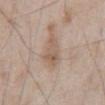Notes:
* workup — catalogued during a skin exam; not biopsied
* anatomic site — the abdomen
* tile lighting — white-light illumination
* patient — male, in their mid- to late 60s
* imaging modality — total-body-photography crop, ~15 mm field of view